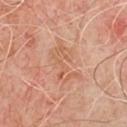The lesion was tiled from a total-body skin photograph and was not biopsied. A male subject in their 50s. Located on the chest. A roughly 15 mm field-of-view crop from a total-body skin photograph.